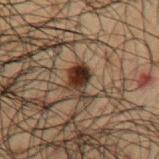* follow-up: total-body-photography surveillance lesion; no biopsy
* diameter: ~3.5 mm (longest diameter)
* lighting: cross-polarized illumination
* location: the left upper arm
* subject: male, in their 50s
* image: 15 mm crop, total-body photography